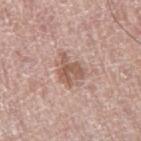Findings:
– workup: total-body-photography surveillance lesion; no biopsy
– location: the right thigh
– image source: total-body-photography crop, ~15 mm field of view
– patient: female, in their 60s